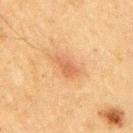Recorded during total-body skin imaging; not selected for excision or biopsy.
A male subject, aged 48 to 52.
The lesion is located on the right upper arm.
Cropped from a total-body skin-imaging series; the visible field is about 15 mm.
About 3 mm across.
Captured under cross-polarized illumination.